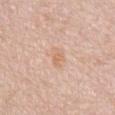Recorded during total-body skin imaging; not selected for excision or biopsy. Imaged with white-light lighting. From the chest. The lesion's longest dimension is about 3 mm. The patient is a male aged around 80. Cropped from a total-body skin-imaging series; the visible field is about 15 mm.